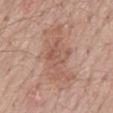This lesion was catalogued during total-body skin photography and was not selected for biopsy.
Automated image analysis of the tile measured an area of roughly 26 mm² and a symmetry-axis asymmetry near 0.25. The software also gave a detector confidence of about 100 out of 100 that the crop contains a lesion.
Located on the mid back.
The tile uses white-light illumination.
A male subject about 70 years old.
Measured at roughly 7.5 mm in maximum diameter.
Cropped from a whole-body photographic skin survey; the tile spans about 15 mm.